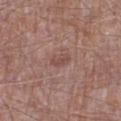{"biopsy_status": "not biopsied; imaged during a skin examination", "lesion_size": {"long_diameter_mm_approx": 2.5}, "image": {"source": "total-body photography crop", "field_of_view_mm": 15}, "automated_metrics": {"area_mm2_approx": 3.0, "eccentricity": 0.85, "shape_asymmetry": 0.3, "vs_skin_darker_L": 7.0, "vs_skin_contrast_norm": 5.5, "peripheral_color_asymmetry": 0.0}, "lighting": "white-light", "patient": {"sex": "male", "age_approx": 65}, "site": "right lower leg"}